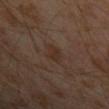| feature | finding |
|---|---|
| image | 15 mm crop, total-body photography |
| patient | male, about 30 years old |
| anatomic site | the left upper arm |
| lesion diameter | ~3 mm (longest diameter) |